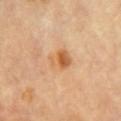The lesion was tiled from a total-body skin photograph and was not biopsied. The lesion is located on the front of the torso. Imaged with cross-polarized lighting. A lesion tile, about 15 mm wide, cut from a 3D total-body photograph. Measured at roughly 3 mm in maximum diameter. A female patient, aged approximately 60.